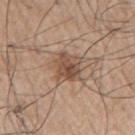• biopsy status · imaged on a skin check; not biopsied